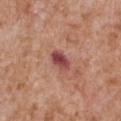Assessment: Part of a total-body skin-imaging series; this lesion was reviewed on a skin check and was not flagged for biopsy. Context: The lesion is located on the chest. This is a white-light tile. A male subject in their mid- to late 60s. The recorded lesion diameter is about 3 mm. A roughly 15 mm field-of-view crop from a total-body skin photograph.